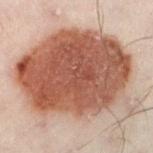A male subject, in their 50s. From the left lower leg. The tile uses cross-polarized illumination. Automated image analysis of the tile measured a lesion area of about 90 mm², an eccentricity of roughly 0.7, and a symmetry-axis asymmetry near 0.2. The analysis additionally found an automated nevus-likeness rating near 100 out of 100. Longest diameter approximately 13.5 mm. A region of skin cropped from a whole-body photographic capture, roughly 15 mm wide.